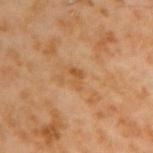Impression:
Recorded during total-body skin imaging; not selected for excision or biopsy.
Clinical summary:
A 15 mm close-up tile from a total-body photography series done for melanoma screening. The tile uses cross-polarized illumination. The lesion's longest dimension is about 2.5 mm. The lesion is on the right upper arm. Automated image analysis of the tile measured a lesion area of about 3 mm², a shape eccentricity near 0.8, and two-axis asymmetry of about 0.45. It also reported an average lesion color of about L≈50 a*≈21 b*≈38 (CIELAB), a lesion–skin lightness drop of about 7, and a lesion-to-skin contrast of about 6 (normalized; higher = more distinct). And it measured a detector confidence of about 100 out of 100 that the crop contains a lesion. The subject is a male about 60 years old.